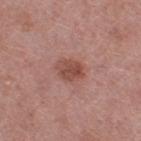Imaged during a routine full-body skin examination; the lesion was not biopsied and no histopathology is available. Approximately 3.5 mm at its widest. A female patient about 70 years old. Located on the right thigh. A 15 mm close-up tile from a total-body photography series done for melanoma screening. Captured under white-light illumination. Automated tile analysis of the lesion measured a footprint of about 6.5 mm² and a symmetry-axis asymmetry near 0.15. The software also gave a mean CIELAB color near L≈49 a*≈24 b*≈25, a lesion–skin lightness drop of about 10, and a normalized lesion–skin contrast near 7.5. The software also gave a nevus-likeness score of about 70/100.